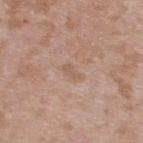follow-up: imaged on a skin check; not biopsied
anatomic site: the upper back
image-analysis metrics: a lesion area of about 2.5 mm², an outline eccentricity of about 0.9 (0 = round, 1 = elongated), and two-axis asymmetry of about 0.4; an average lesion color of about L≈57 a*≈18 b*≈29 (CIELAB), roughly 6 lightness units darker than nearby skin, and a normalized border contrast of about 5; a classifier nevus-likeness of about 0/100 and a lesion-detection confidence of about 100/100
subject: male, aged around 35
acquisition: ~15 mm tile from a whole-body skin photo
size: ~2.5 mm (longest diameter)